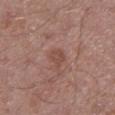biopsy status: no biopsy performed (imaged during a skin exam) | lighting: white-light illumination | image: 15 mm crop, total-body photography | site: the left lower leg | subject: male, aged 43–47 | size: ≈2.5 mm.